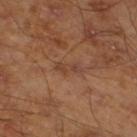Impression:
Captured during whole-body skin photography for melanoma surveillance; the lesion was not biopsied.
Clinical summary:
A 15 mm close-up extracted from a 3D total-body photography capture. The lesion is on the left lower leg. The lesion-visualizer software estimated an average lesion color of about L≈41 a*≈21 b*≈29 (CIELAB). And it measured a within-lesion color-variation index near 0.5/10 and a peripheral color-asymmetry measure near 0. It also reported a classifier nevus-likeness of about 0/100 and a detector confidence of about 100 out of 100 that the crop contains a lesion. The lesion's longest dimension is about 3 mm. A male patient, in their mid- to late 40s.